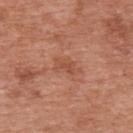Clinical impression:
This lesion was catalogued during total-body skin photography and was not selected for biopsy.
Acquisition and patient details:
A male patient, aged approximately 70. An algorithmic analysis of the crop reported a lesion–skin lightness drop of about 7 and a lesion-to-skin contrast of about 5.5 (normalized; higher = more distinct). The analysis additionally found an automated nevus-likeness rating near 0 out of 100 and lesion-presence confidence of about 100/100. The lesion is on the upper back. Measured at roughly 3 mm in maximum diameter. Cropped from a total-body skin-imaging series; the visible field is about 15 mm. Captured under white-light illumination.